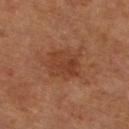{
  "biopsy_status": "not biopsied; imaged during a skin examination",
  "patient": {
    "sex": "female",
    "age_approx": 65
  },
  "lighting": "cross-polarized",
  "site": "left lower leg",
  "image": {
    "source": "total-body photography crop",
    "field_of_view_mm": 15
  }
}